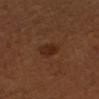Assessment: Part of a total-body skin-imaging series; this lesion was reviewed on a skin check and was not flagged for biopsy. Context: Longest diameter approximately 2.5 mm. On the arm. Automated tile analysis of the lesion measured a lesion area of about 4.5 mm², an outline eccentricity of about 0.6 (0 = round, 1 = elongated), and a symmetry-axis asymmetry near 0.25. It also reported an average lesion color of about L≈26 a*≈20 b*≈27 (CIELAB), about 7 CIELAB-L* units darker than the surrounding skin, and a normalized border contrast of about 7.5. The subject is a male approximately 50 years of age. Captured under cross-polarized illumination. Cropped from a total-body skin-imaging series; the visible field is about 15 mm.